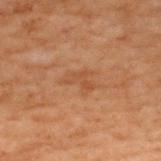Captured during whole-body skin photography for melanoma surveillance; the lesion was not biopsied.